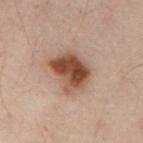Clinical impression:
Captured during whole-body skin photography for melanoma surveillance; the lesion was not biopsied.
Image and clinical context:
A female patient, in their mid- to late 50s. The tile uses cross-polarized illumination. Located on the abdomen. The lesion's longest dimension is about 4.5 mm. A roughly 15 mm field-of-view crop from a total-body skin photograph.